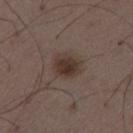The tile uses white-light illumination.
Cropped from a whole-body photographic skin survey; the tile spans about 15 mm.
Located on the left thigh.
Measured at roughly 3 mm in maximum diameter.
A male patient aged 48 to 52.